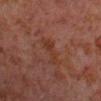Captured during whole-body skin photography for melanoma surveillance; the lesion was not biopsied.
This image is a 15 mm lesion crop taken from a total-body photograph.
An algorithmic analysis of the crop reported an outline eccentricity of about 0.9 (0 = round, 1 = elongated). It also reported a mean CIELAB color near L≈29 a*≈19 b*≈24, about 4 CIELAB-L* units darker than the surrounding skin, and a normalized border contrast of about 6. And it measured a color-variation rating of about 1/10. And it measured a nevus-likeness score of about 0/100 and lesion-presence confidence of about 100/100.
The subject is a male about 30 years old.
The recorded lesion diameter is about 3.5 mm.
The lesion is located on the right forearm.
Imaged with cross-polarized lighting.